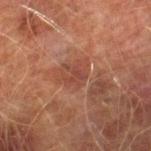• subject: male, about 75 years old
• image source: total-body-photography crop, ~15 mm field of view
• location: the right lower leg
• lesion size: ~2.5 mm (longest diameter)